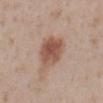A female subject in their 30s.
From the mid back.
A roughly 15 mm field-of-view crop from a total-body skin photograph.